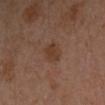Recorded during total-body skin imaging; not selected for excision or biopsy. A 15 mm close-up extracted from a 3D total-body photography capture. The lesion is on the left forearm. A female subject, aged around 40. The total-body-photography lesion software estimated an area of roughly 4.5 mm² and an outline eccentricity of about 0.75 (0 = round, 1 = elongated). The software also gave roughly 6 lightness units darker than nearby skin. And it measured a border-irregularity index near 2/10 and a peripheral color-asymmetry measure near 0.5. Imaged with cross-polarized lighting. Longest diameter approximately 3 mm.